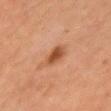No biopsy was performed on this lesion — it was imaged during a full skin examination and was not determined to be concerning. The lesion-visualizer software estimated a shape-asymmetry score of about 0.2 (0 = symmetric). It also reported an average lesion color of about L≈40 a*≈22 b*≈30 (CIELAB), about 10 CIELAB-L* units darker than the surrounding skin, and a normalized border contrast of about 8.5. The patient is a female aged 58–62. Cropped from a total-body skin-imaging series; the visible field is about 15 mm. Located on the left upper arm. About 2.5 mm across. Imaged with cross-polarized lighting.